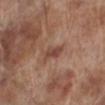The lesion was tiled from a total-body skin photograph and was not biopsied. The recorded lesion diameter is about 3 mm. The tile uses white-light illumination. Cropped from a total-body skin-imaging series; the visible field is about 15 mm. Automated tile analysis of the lesion measured a footprint of about 4 mm², an outline eccentricity of about 0.85 (0 = round, 1 = elongated), and a shape-asymmetry score of about 0.35 (0 = symmetric). The analysis additionally found a border-irregularity index near 3.5/10 and internal color variation of about 3 on a 0–10 scale. A male subject about 70 years old. The lesion is on the left lower leg.